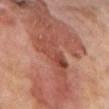Findings:
- notes · no biopsy performed (imaged during a skin exam)
- site · the leg
- illumination · cross-polarized illumination
- acquisition · total-body-photography crop, ~15 mm field of view
- lesion size · about 5 mm
- subject · male, aged 68 to 72